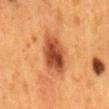workup = catalogued during a skin exam; not biopsied
image source = total-body-photography crop, ~15 mm field of view
patient = female, aged 48–52
lighting = cross-polarized
site = the mid back
diameter = ~5.5 mm (longest diameter)